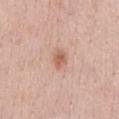biopsy status = imaged on a skin check; not biopsied
location = the chest
lesion diameter = ~2.5 mm (longest diameter)
imaging modality = total-body-photography crop, ~15 mm field of view
patient = female, approximately 40 years of age
lighting = white-light illumination